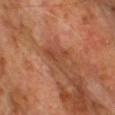{"biopsy_status": "not biopsied; imaged during a skin examination", "site": "upper back", "image": {"source": "total-body photography crop", "field_of_view_mm": 15}, "patient": {"sex": "male", "age_approx": 75}}